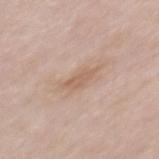– biopsy status · imaged on a skin check; not biopsied
– image source · 15 mm crop, total-body photography
– illumination · white-light
– anatomic site · the back
– subject · female, approximately 30 years of age
– automated lesion analysis · an average lesion color of about L≈60 a*≈18 b*≈29 (CIELAB), about 8 CIELAB-L* units darker than the surrounding skin, and a normalized lesion–skin contrast near 5.5; a classifier nevus-likeness of about 0/100 and a detector confidence of about 100 out of 100 that the crop contains a lesion